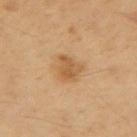Assessment:
Part of a total-body skin-imaging series; this lesion was reviewed on a skin check and was not flagged for biopsy.
Acquisition and patient details:
A male subject about 45 years old. On the right upper arm. A 15 mm crop from a total-body photograph taken for skin-cancer surveillance.